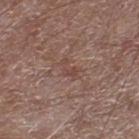Part of a total-body skin-imaging series; this lesion was reviewed on a skin check and was not flagged for biopsy.
A region of skin cropped from a whole-body photographic capture, roughly 15 mm wide.
The lesion's longest dimension is about 2.5 mm.
On the right lower leg.
The patient is a male aged around 70.
Automated tile analysis of the lesion measured an area of roughly 3.5 mm² and a symmetry-axis asymmetry near 0.5. It also reported an average lesion color of about L≈45 a*≈19 b*≈23 (CIELAB), roughly 6 lightness units darker than nearby skin, and a normalized lesion–skin contrast near 5. And it measured border irregularity of about 6 on a 0–10 scale, a within-lesion color-variation index near 0.5/10, and peripheral color asymmetry of about 0. The software also gave an automated nevus-likeness rating near 0 out of 100 and lesion-presence confidence of about 100/100.
This is a white-light tile.